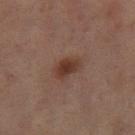The lesion was photographed on a routine skin check and not biopsied; there is no pathology result.
A 15 mm crop from a total-body photograph taken for skin-cancer surveillance.
On the right thigh.
The patient is a female aged around 60.